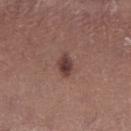Recorded during total-body skin imaging; not selected for excision or biopsy. Automated image analysis of the tile measured a classifier nevus-likeness of about 90/100 and a lesion-detection confidence of about 100/100. Imaged with white-light lighting. About 2.5 mm across. From the left lower leg. This image is a 15 mm lesion crop taken from a total-body photograph. A female subject, approximately 20 years of age.